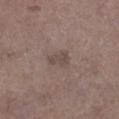biopsy status=total-body-photography surveillance lesion; no biopsy
imaging modality=15 mm crop, total-body photography
body site=the right lower leg
illumination=white-light
patient=female, about 65 years old
diameter=about 2.5 mm